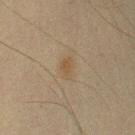No biopsy was performed on this lesion — it was imaged during a full skin examination and was not determined to be concerning.
Located on the left upper arm.
This is a cross-polarized tile.
This image is a 15 mm lesion crop taken from a total-body photograph.
A male patient aged approximately 35.
The recorded lesion diameter is about 2.5 mm.